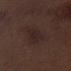No biopsy was performed on this lesion — it was imaged during a full skin examination and was not determined to be concerning. This is a white-light tile. The lesion's longest dimension is about 6 mm. A close-up tile cropped from a whole-body skin photograph, about 15 mm across. On the left upper arm. A male patient in their 70s.